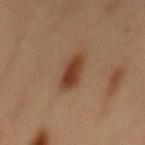{"biopsy_status": "not biopsied; imaged during a skin examination", "lighting": "cross-polarized", "image": {"source": "total-body photography crop", "field_of_view_mm": 15}, "site": "front of the torso", "patient": {"sex": "male", "age_approx": 70}}